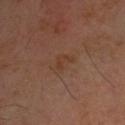<case>
<image>
  <source>total-body photography crop</source>
  <field_of_view_mm>15</field_of_view_mm>
</image>
<patient>
  <sex>male</sex>
  <age_approx>70</age_approx>
</patient>
<site>head or neck</site>
<lighting>cross-polarized</lighting>
</case>